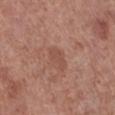Impression:
This lesion was catalogued during total-body skin photography and was not selected for biopsy.
Image and clinical context:
Longest diameter approximately 3 mm. An algorithmic analysis of the crop reported a lesion area of about 3.5 mm², a shape eccentricity near 0.85, and a shape-asymmetry score of about 0.4 (0 = symmetric). The software also gave a mean CIELAB color near L≈49 a*≈24 b*≈26, a lesion–skin lightness drop of about 6, and a normalized border contrast of about 4.5. The analysis additionally found a border-irregularity rating of about 3.5/10 and radial color variation of about 0.5. And it measured a classifier nevus-likeness of about 0/100 and lesion-presence confidence of about 100/100. Located on the right lower leg. This is a white-light tile. A male subject aged 53 to 57. A 15 mm close-up extracted from a 3D total-body photography capture.